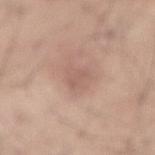Q: Is there a histopathology result?
A: imaged on a skin check; not biopsied
Q: Where on the body is the lesion?
A: the back
Q: Patient demographics?
A: male, about 35 years old
Q: Illumination type?
A: white-light illumination
Q: How large is the lesion?
A: about 3 mm
Q: What is the imaging modality?
A: total-body-photography crop, ~15 mm field of view